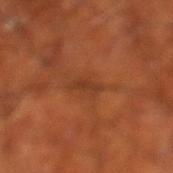Part of a total-body skin-imaging series; this lesion was reviewed on a skin check and was not flagged for biopsy. About 3.5 mm across. A region of skin cropped from a whole-body photographic capture, roughly 15 mm wide. Imaged with cross-polarized lighting. On the left lower leg. Automated tile analysis of the lesion measured a classifier nevus-likeness of about 0/100 and a detector confidence of about 65 out of 100 that the crop contains a lesion. A male patient, aged approximately 70.